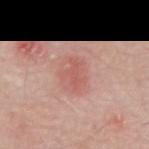The lesion was photographed on a routine skin check and not biopsied; there is no pathology result. A male subject roughly 70 years of age. The lesion is located on the mid back. Imaged with white-light lighting. A 15 mm close-up tile from a total-body photography series done for melanoma screening.